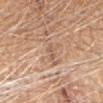<tbp_lesion>
  <biopsy_status>not biopsied; imaged during a skin examination</biopsy_status>
  <image>
    <source>total-body photography crop</source>
    <field_of_view_mm>15</field_of_view_mm>
  </image>
  <patient>
    <sex>female</sex>
    <age_approx>70</age_approx>
  </patient>
  <lesion_size>
    <long_diameter_mm_approx>2.5</long_diameter_mm_approx>
  </lesion_size>
  <lighting>white-light</lighting>
  <site>head or neck</site>
</tbp_lesion>